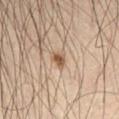Notes:
- notes · catalogued during a skin exam; not biopsied
- patient · male, aged approximately 65
- image-analysis metrics · a footprint of about 2.5 mm²; a classifier nevus-likeness of about 95/100 and lesion-presence confidence of about 100/100
- anatomic site · the right thigh
- diameter · ~2 mm (longest diameter)
- tile lighting · cross-polarized
- image · 15 mm crop, total-body photography